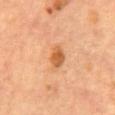Captured during whole-body skin photography for melanoma surveillance; the lesion was not biopsied.
The patient is a female in their 60s.
The recorded lesion diameter is about 3.5 mm.
This is a cross-polarized tile.
From the chest.
Cropped from a whole-body photographic skin survey; the tile spans about 15 mm.
Automated image analysis of the tile measured about 11 CIELAB-L* units darker than the surrounding skin. The software also gave an automated nevus-likeness rating near 85 out of 100.